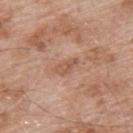The lesion is on the upper back.
A male subject aged approximately 55.
An algorithmic analysis of the crop reported a border-irregularity index near 3.5/10, a color-variation rating of about 1.5/10, and a peripheral color-asymmetry measure near 0.5.
Measured at roughly 3 mm in maximum diameter.
Cropped from a whole-body photographic skin survey; the tile spans about 15 mm.